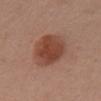{"biopsy_status": "not biopsied; imaged during a skin examination", "patient": {"sex": "male", "age_approx": 40}, "automated_metrics": {"border_irregularity_0_10": 1.5, "color_variation_0_10": 3.5, "peripheral_color_asymmetry": 1.0, "nevus_likeness_0_100": 100}, "site": "chest", "image": {"source": "total-body photography crop", "field_of_view_mm": 15}, "lesion_size": {"long_diameter_mm_approx": 5.0}, "lighting": "white-light"}